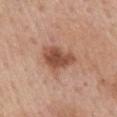Q: Was a biopsy performed?
A: catalogued during a skin exam; not biopsied
Q: Illumination type?
A: white-light
Q: Lesion location?
A: the mid back
Q: What kind of image is this?
A: ~15 mm tile from a whole-body skin photo
Q: Patient demographics?
A: female, roughly 65 years of age
Q: Automated lesion metrics?
A: a mean CIELAB color near L≈51 a*≈23 b*≈30, a lesion–skin lightness drop of about 13, and a lesion-to-skin contrast of about 8.5 (normalized; higher = more distinct)